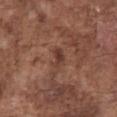Clinical summary:
Automated image analysis of the tile measured about 8 CIELAB-L* units darker than the surrounding skin and a normalized border contrast of about 7. It also reported a border-irregularity index near 2.5/10, a within-lesion color-variation index near 3.5/10, and radial color variation of about 1.5. The software also gave a detector confidence of about 100 out of 100 that the crop contains a lesion. The lesion is located on the abdomen. Approximately 2.5 mm at its widest. Imaged with white-light lighting. A 15 mm close-up tile from a total-body photography series done for melanoma screening. The patient is a male roughly 75 years of age.